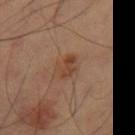Part of a total-body skin-imaging series; this lesion was reviewed on a skin check and was not flagged for biopsy. An algorithmic analysis of the crop reported a border-irregularity rating of about 2.5/10, internal color variation of about 4 on a 0–10 scale, and peripheral color asymmetry of about 1.5. And it measured lesion-presence confidence of about 100/100. The lesion's longest dimension is about 3 mm. A 15 mm close-up extracted from a 3D total-body photography capture. On the leg.